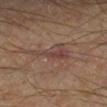workup = catalogued during a skin exam; not biopsied
tile lighting = cross-polarized
location = the right lower leg
subject = male, aged 68–72
lesion size = ≈5.5 mm
acquisition = 15 mm crop, total-body photography